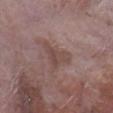biopsy_status: not biopsied; imaged during a skin examination
lesion_size:
  long_diameter_mm_approx: 4.0
automated_metrics:
  area_mm2_approx: 7.5
  eccentricity: 0.65
  border_irregularity_0_10: 6.0
  color_variation_0_10: 3.0
  peripheral_color_asymmetry: 1.0
  nevus_likeness_0_100: 0
patient:
  sex: male
  age_approx: 75
image:
  source: total-body photography crop
  field_of_view_mm: 15
site: right lower leg